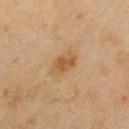Impression: The lesion was tiled from a total-body skin photograph and was not biopsied. Acquisition and patient details: On the left upper arm. A close-up tile cropped from a whole-body skin photograph, about 15 mm across. The subject is a male aged approximately 45.